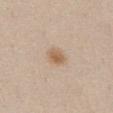Impression: The lesion was tiled from a total-body skin photograph and was not biopsied.